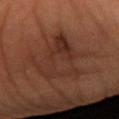Part of a total-body skin-imaging series; this lesion was reviewed on a skin check and was not flagged for biopsy.
Measured at roughly 6.5 mm in maximum diameter.
A close-up tile cropped from a whole-body skin photograph, about 15 mm across.
An algorithmic analysis of the crop reported an area of roughly 21 mm², an eccentricity of roughly 0.7, and two-axis asymmetry of about 0.45. And it measured an average lesion color of about L≈31 a*≈21 b*≈26 (CIELAB), about 7 CIELAB-L* units darker than the surrounding skin, and a normalized lesion–skin contrast near 6.5. The software also gave border irregularity of about 7.5 on a 0–10 scale, a color-variation rating of about 5.5/10, and a peripheral color-asymmetry measure near 2. It also reported an automated nevus-likeness rating near 0 out of 100.
Located on the right forearm.
A female subject in their 40s.